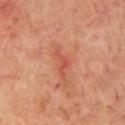A region of skin cropped from a whole-body photographic capture, roughly 15 mm wide. The patient is a male aged 68–72. Imaged with cross-polarized lighting. Approximately 3.5 mm at its widest. The total-body-photography lesion software estimated a lesion area of about 3.5 mm² and a symmetry-axis asymmetry near 0.6. And it measured an average lesion color of about L≈51 a*≈30 b*≈33 (CIELAB), about 8 CIELAB-L* units darker than the surrounding skin, and a lesion-to-skin contrast of about 5.5 (normalized; higher = more distinct). The analysis additionally found a classifier nevus-likeness of about 0/100 and a detector confidence of about 100 out of 100 that the crop contains a lesion. The lesion is on the chest.